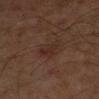Clinical impression:
The lesion was tiled from a total-body skin photograph and was not biopsied.
Background:
A 15 mm close-up tile from a total-body photography series done for melanoma screening. The lesion is on the right lower leg. The lesion-visualizer software estimated a border-irregularity index near 3.5/10 and a peripheral color-asymmetry measure near 1.5. The software also gave a classifier nevus-likeness of about 25/100 and a lesion-detection confidence of about 100/100. The tile uses cross-polarized illumination. The subject is a male aged 58–62.